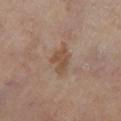Clinical impression:
Part of a total-body skin-imaging series; this lesion was reviewed on a skin check and was not flagged for biopsy.
Image and clinical context:
A close-up tile cropped from a whole-body skin photograph, about 15 mm across. The lesion's longest dimension is about 3.5 mm. A male subject, aged approximately 65. The lesion is located on the left lower leg.